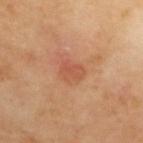Case summary:
- biopsy status: imaged on a skin check; not biopsied
- lesion size: about 2.5 mm
- subject: female, aged 63 to 67
- anatomic site: the upper back
- imaging modality: ~15 mm crop, total-body skin-cancer survey